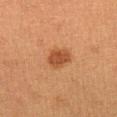biopsy_status: not biopsied; imaged during a skin examination
patient:
  sex: female
  age_approx: 40
site: right lower leg
image:
  source: total-body photography crop
  field_of_view_mm: 15